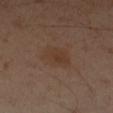workup: no biopsy performed (imaged during a skin exam)
patient: male, in their mid- to late 50s
image: 15 mm crop, total-body photography
TBP lesion metrics: a footprint of about 9 mm², a shape eccentricity near 0.7, and two-axis asymmetry of about 0.2; an average lesion color of about L≈36 a*≈16 b*≈26 (CIELAB) and a normalized border contrast of about 5.5
lighting: cross-polarized illumination
lesion diameter: ~4 mm (longest diameter)
location: the left forearm